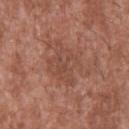follow-up=no biopsy performed (imaged during a skin exam) | body site=the upper back | subject=male, roughly 45 years of age | lesion diameter=≈5.5 mm | image=15 mm crop, total-body photography | automated metrics=an area of roughly 13 mm², an eccentricity of roughly 0.65, and two-axis asymmetry of about 0.3; a border-irregularity rating of about 5/10 and peripheral color asymmetry of about 1; an automated nevus-likeness rating near 0 out of 100 and a detector confidence of about 100 out of 100 that the crop contains a lesion | tile lighting=white-light illumination.